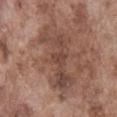No biopsy was performed on this lesion — it was imaged during a full skin examination and was not determined to be concerning.
On the abdomen.
A male patient in their mid- to late 70s.
The tile uses white-light illumination.
Automated tile analysis of the lesion measured a lesion area of about 18 mm², an outline eccentricity of about 0.9 (0 = round, 1 = elongated), and a symmetry-axis asymmetry near 0.45. And it measured a within-lesion color-variation index near 4/10 and a peripheral color-asymmetry measure near 1.
About 8.5 mm across.
Cropped from a total-body skin-imaging series; the visible field is about 15 mm.